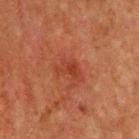No biopsy was performed on this lesion — it was imaged during a full skin examination and was not determined to be concerning. A male subject, in their mid- to late 60s. The lesion is on the front of the torso. Approximately 3 mm at its widest. A 15 mm close-up extracted from a 3D total-body photography capture. The total-body-photography lesion software estimated an average lesion color of about L≈33 a*≈28 b*≈29 (CIELAB), about 6 CIELAB-L* units darker than the surrounding skin, and a normalized lesion–skin contrast near 6. The analysis additionally found border irregularity of about 5 on a 0–10 scale, internal color variation of about 1.5 on a 0–10 scale, and radial color variation of about 0.5. And it measured a nevus-likeness score of about 10/100 and lesion-presence confidence of about 100/100. Imaged with cross-polarized lighting.